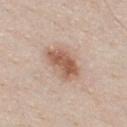| field | value |
|---|---|
| follow-up | catalogued during a skin exam; not biopsied |
| body site | the chest |
| lesion size | ~4.5 mm (longest diameter) |
| patient | male, aged approximately 45 |
| image source | 15 mm crop, total-body photography |
| automated lesion analysis | a shape eccentricity near 0.85 and a shape-asymmetry score of about 0.3 (0 = symmetric); a normalized border contrast of about 8.5 |